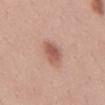Clinical impression:
This lesion was catalogued during total-body skin photography and was not selected for biopsy.
Clinical summary:
The total-body-photography lesion software estimated an eccentricity of roughly 0.85 and a shape-asymmetry score of about 0.25 (0 = symmetric). And it measured a lesion color around L≈57 a*≈23 b*≈28 in CIELAB and a lesion-to-skin contrast of about 7.5 (normalized; higher = more distinct). And it measured a border-irregularity rating of about 3/10, a within-lesion color-variation index near 3/10, and a peripheral color-asymmetry measure near 1. A female patient aged 33–37. The lesion is on the back. A region of skin cropped from a whole-body photographic capture, roughly 15 mm wide.